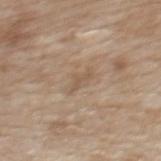biopsy status — no biopsy performed (imaged during a skin exam); imaging modality — 15 mm crop, total-body photography; patient — female, aged 38–42; anatomic site — the upper back.